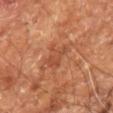Impression: No biopsy was performed on this lesion — it was imaged during a full skin examination and was not determined to be concerning. Context: A male patient aged around 60. The lesion is on the right leg. This is a cross-polarized tile. A 15 mm crop from a total-body photograph taken for skin-cancer surveillance. Measured at roughly 3.5 mm in maximum diameter. An algorithmic analysis of the crop reported a shape eccentricity near 0.6 and two-axis asymmetry of about 0.3. The software also gave an average lesion color of about L≈47 a*≈26 b*≈34 (CIELAB), about 7 CIELAB-L* units darker than the surrounding skin, and a normalized lesion–skin contrast near 5.5. And it measured an automated nevus-likeness rating near 0 out of 100 and a lesion-detection confidence of about 100/100.